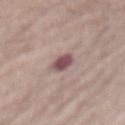Imaged during a routine full-body skin examination; the lesion was not biopsied and no histopathology is available.
Cropped from a total-body skin-imaging series; the visible field is about 15 mm.
Located on the abdomen.
The recorded lesion diameter is about 3 mm.
A female patient aged around 65.
The tile uses white-light illumination.